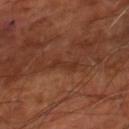follow-up: total-body-photography surveillance lesion; no biopsy | acquisition: ~15 mm tile from a whole-body skin photo | size: ≈3.5 mm | illumination: cross-polarized | subject: aged 63 to 67 | location: the right lower leg.